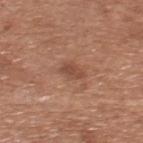Impression: No biopsy was performed on this lesion — it was imaged during a full skin examination and was not determined to be concerning. Acquisition and patient details: A male patient, approximately 65 years of age. On the upper back. This image is a 15 mm lesion crop taken from a total-body photograph.